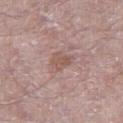Q: Is there a histopathology result?
A: total-body-photography surveillance lesion; no biopsy
Q: What are the patient's age and sex?
A: male, aged around 65
Q: Illumination type?
A: white-light
Q: What is the anatomic site?
A: the right thigh
Q: What kind of image is this?
A: 15 mm crop, total-body photography